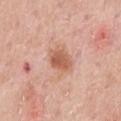The lesion was photographed on a routine skin check and not biopsied; there is no pathology result. The lesion is on the chest. A 15 mm crop from a total-body photograph taken for skin-cancer surveillance. The patient is a male in their 60s. Longest diameter approximately 3.5 mm. The lesion-visualizer software estimated an area of roughly 9 mm² and a symmetry-axis asymmetry near 0.15. The software also gave about 10 CIELAB-L* units darker than the surrounding skin and a lesion-to-skin contrast of about 7.5 (normalized; higher = more distinct).